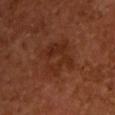The lesion was photographed on a routine skin check and not biopsied; there is no pathology result. Cropped from a whole-body photographic skin survey; the tile spans about 15 mm. About 5 mm across. The lesion-visualizer software estimated an average lesion color of about L≈26 a*≈21 b*≈27 (CIELAB), roughly 5 lightness units darker than nearby skin, and a normalized border contrast of about 6. It also reported border irregularity of about 4 on a 0–10 scale, internal color variation of about 3.5 on a 0–10 scale, and a peripheral color-asymmetry measure near 1. Imaged with cross-polarized lighting. The lesion is on the back. A male subject, aged around 55.